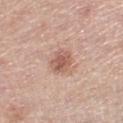<lesion>
<biopsy_status>not biopsied; imaged during a skin examination</biopsy_status>
<patient>
  <sex>female</sex>
  <age_approx>65</age_approx>
</patient>
<lesion_size>
  <long_diameter_mm_approx>3.0</long_diameter_mm_approx>
</lesion_size>
<site>right lower leg</site>
<image>
  <source>total-body photography crop</source>
  <field_of_view_mm>15</field_of_view_mm>
</image>
<lighting>white-light</lighting>
</lesion>